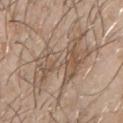The lesion was tiled from a total-body skin photograph and was not biopsied.
Longest diameter approximately 8 mm.
The lesion-visualizer software estimated an eccentricity of roughly 0.8. The analysis additionally found a mean CIELAB color near L≈54 a*≈14 b*≈27, about 8 CIELAB-L* units darker than the surrounding skin, and a lesion-to-skin contrast of about 5.5 (normalized; higher = more distinct). The software also gave a classifier nevus-likeness of about 0/100 and a detector confidence of about 60 out of 100 that the crop contains a lesion.
This image is a 15 mm lesion crop taken from a total-body photograph.
Imaged with white-light lighting.
On the chest.
The subject is a male approximately 30 years of age.